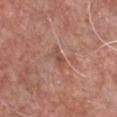site: chest
patient:
  sex: male
  age_approx: 55
automated_metrics:
  area_mm2_approx: 3.0
  eccentricity: 0.85
  shape_asymmetry: 0.4
  cielab_L: 50
  cielab_a: 24
  cielab_b: 29
  color_variation_0_10: 2.0
  peripheral_color_asymmetry: 0.5
image:
  source: total-body photography crop
  field_of_view_mm: 15